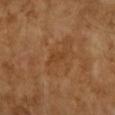Notes:
– follow-up · imaged on a skin check; not biopsied
– location · the right forearm
– illumination · cross-polarized
– acquisition · 15 mm crop, total-body photography
– patient · female, approximately 70 years of age
– automated lesion analysis · an area of roughly 2.5 mm², an outline eccentricity of about 0.9 (0 = round, 1 = elongated), and a symmetry-axis asymmetry near 0.35; an average lesion color of about L≈42 a*≈22 b*≈37 (CIELAB) and about 6 CIELAB-L* units darker than the surrounding skin; a color-variation rating of about 0/10; a nevus-likeness score of about 0/100 and lesion-presence confidence of about 100/100